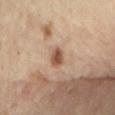workup = total-body-photography surveillance lesion; no biopsy | diameter = ~2.5 mm (longest diameter) | site = the back | automated metrics = a lesion area of about 4 mm², a shape eccentricity near 0.7, and two-axis asymmetry of about 0.25; a border-irregularity rating of about 2.5/10 and internal color variation of about 2.5 on a 0–10 scale; a classifier nevus-likeness of about 80/100 and a lesion-detection confidence of about 100/100 | tile lighting = cross-polarized | subject = female, about 60 years old | image source = ~15 mm crop, total-body skin-cancer survey.